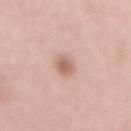Recorded during total-body skin imaging; not selected for excision or biopsy.
The patient is a female aged around 70.
A 15 mm crop from a total-body photograph taken for skin-cancer surveillance.
The lesion is on the mid back.